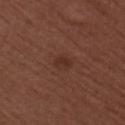Clinical impression:
No biopsy was performed on this lesion — it was imaged during a full skin examination and was not determined to be concerning.
Acquisition and patient details:
Located on the arm. Captured under white-light illumination. The lesion's longest dimension is about 2 mm. Cropped from a whole-body photographic skin survey; the tile spans about 15 mm. Automated image analysis of the tile measured a footprint of about 2 mm², an eccentricity of roughly 0.85, and a shape-asymmetry score of about 0.3 (0 = symmetric). It also reported a classifier nevus-likeness of about 35/100. A female subject, aged around 50.